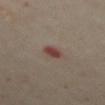Recorded during total-body skin imaging; not selected for excision or biopsy.
The subject is a female in their 60s.
The tile uses cross-polarized illumination.
Cropped from a total-body skin-imaging series; the visible field is about 15 mm.
Measured at roughly 2.5 mm in maximum diameter.
On the abdomen.